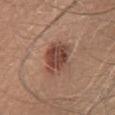{"biopsy_status": "not biopsied; imaged during a skin examination", "site": "chest", "lesion_size": {"long_diameter_mm_approx": 4.5}, "lighting": "white-light", "automated_metrics": {"area_mm2_approx": 12.0, "eccentricity": 0.7, "shape_asymmetry": 0.2}, "patient": {"sex": "male", "age_approx": 65}, "image": {"source": "total-body photography crop", "field_of_view_mm": 15}}